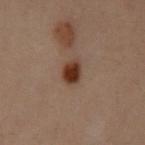Impression:
The lesion was photographed on a routine skin check and not biopsied; there is no pathology result.
Background:
A female subject, aged 28–32. This is a cross-polarized tile. The lesion is located on the left upper arm. A close-up tile cropped from a whole-body skin photograph, about 15 mm across. The lesion-visualizer software estimated a shape eccentricity near 0.5 and a symmetry-axis asymmetry near 0.15. The analysis additionally found a border-irregularity rating of about 1.5/10, a color-variation rating of about 3.5/10, and a peripheral color-asymmetry measure near 1. It also reported a nevus-likeness score of about 100/100 and lesion-presence confidence of about 100/100.